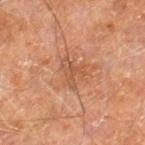Impression: The lesion was photographed on a routine skin check and not biopsied; there is no pathology result. Clinical summary: This is a cross-polarized tile. A male subject approximately 60 years of age. The lesion is on the right leg. About 3.5 mm across. Cropped from a total-body skin-imaging series; the visible field is about 15 mm. Automated tile analysis of the lesion measured an eccentricity of roughly 0.6 and a symmetry-axis asymmetry near 0.65. The software also gave a border-irregularity rating of about 7/10 and radial color variation of about 0.5.